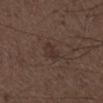Findings:
• biopsy status · total-body-photography surveillance lesion; no biopsy
• image source · total-body-photography crop, ~15 mm field of view
• tile lighting · white-light
• patient · male, aged 48–52
• TBP lesion metrics · a lesion area of about 3.5 mm², a shape eccentricity near 0.8, and a symmetry-axis asymmetry near 0.3; a lesion color around L≈31 a*≈14 b*≈20 in CIELAB, about 5 CIELAB-L* units darker than the surrounding skin, and a normalized border contrast of about 5.5; a nevus-likeness score of about 5/100 and a detector confidence of about 100 out of 100 that the crop contains a lesion
• body site · the right lower leg